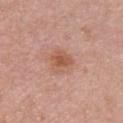Findings:
* biopsy status: no biopsy performed (imaged during a skin exam)
* lighting: white-light illumination
* patient: female, about 30 years old
* site: the chest
* size: ≈3 mm
* image: ~15 mm tile from a whole-body skin photo